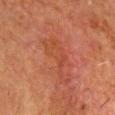This lesion was catalogued during total-body skin photography and was not selected for biopsy.
Automated image analysis of the tile measured a classifier nevus-likeness of about 0/100 and a lesion-detection confidence of about 100/100.
Measured at roughly 5.5 mm in maximum diameter.
A male subject roughly 80 years of age.
A lesion tile, about 15 mm wide, cut from a 3D total-body photograph.
The lesion is on the head or neck.